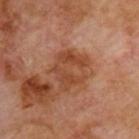{
  "site": "back",
  "image": {
    "source": "total-body photography crop",
    "field_of_view_mm": 15
  },
  "automated_metrics": {
    "vs_skin_contrast_norm": 7.0,
    "border_irregularity_0_10": 4.0,
    "peripheral_color_asymmetry": 1.5
  },
  "lesion_size": {
    "long_diameter_mm_approx": 5.0
  },
  "lighting": "cross-polarized",
  "patient": {
    "sex": "male",
    "age_approx": 70
  }
}